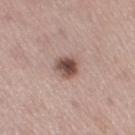Impression:
Recorded during total-body skin imaging; not selected for excision or biopsy.
Context:
A female subject, aged 48 to 52. About 3 mm across. Cropped from a whole-body photographic skin survey; the tile spans about 15 mm. The lesion is located on the right thigh. The total-body-photography lesion software estimated a footprint of about 5.5 mm². This is a white-light tile.